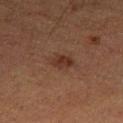The lesion was tiled from a total-body skin photograph and was not biopsied. A 15 mm close-up tile from a total-body photography series done for melanoma screening. A male subject in their mid-70s. The lesion-visualizer software estimated a nevus-likeness score of about 65/100 and lesion-presence confidence of about 100/100. The lesion is on the leg. Approximately 3.5 mm at its widest. Captured under cross-polarized illumination.